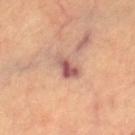The lesion was photographed on a routine skin check and not biopsied; there is no pathology result.